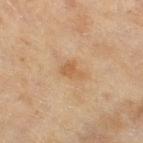workup = imaged on a skin check; not biopsied
acquisition = total-body-photography crop, ~15 mm field of view
patient = female, aged around 60
body site = the right thigh